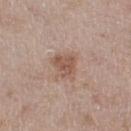Findings:
• follow-up — imaged on a skin check; not biopsied
• anatomic site — the right thigh
• size — about 4 mm
• subject — female, aged 58 to 62
• illumination — white-light illumination
• acquisition — ~15 mm tile from a whole-body skin photo